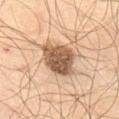workup = total-body-photography surveillance lesion; no biopsy
anatomic site = the abdomen
acquisition = 15 mm crop, total-body photography
subject = male, in their mid-50s